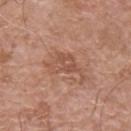{"biopsy_status": "not biopsied; imaged during a skin examination", "patient": {"sex": "male", "age_approx": 65}, "image": {"source": "total-body photography crop", "field_of_view_mm": 15}, "lighting": "white-light", "site": "chest", "automated_metrics": {"border_irregularity_0_10": 6.5, "color_variation_0_10": 3.0, "peripheral_color_asymmetry": 1.0, "nevus_likeness_0_100": 0, "lesion_detection_confidence_0_100": 100}, "lesion_size": {"long_diameter_mm_approx": 5.0}}